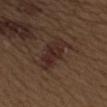follow-up: catalogued during a skin exam; not biopsied
body site: the right upper arm
subject: male, about 70 years old
illumination: white-light
diameter: ~4.5 mm (longest diameter)
acquisition: ~15 mm crop, total-body skin-cancer survey